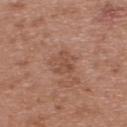<record>
  <patient>
    <sex>female</sex>
    <age_approx>40</age_approx>
  </patient>
  <site>upper back</site>
  <image>
    <source>total-body photography crop</source>
    <field_of_view_mm>15</field_of_view_mm>
  </image>
  <lesion_size>
    <long_diameter_mm_approx>2.5</long_diameter_mm_approx>
  </lesion_size>
  <lighting>white-light</lighting>
</record>